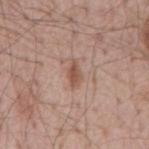• biopsy status: total-body-photography surveillance lesion; no biopsy
• tile lighting: white-light illumination
• subject: male, aged approximately 55
• site: the mid back
• image: 15 mm crop, total-body photography